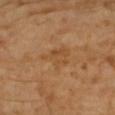The lesion was photographed on a routine skin check and not biopsied; there is no pathology result. The lesion is located on the right upper arm. A close-up tile cropped from a whole-body skin photograph, about 15 mm across. The subject is a female roughly 70 years of age.